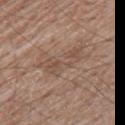Assessment: The lesion was photographed on a routine skin check and not biopsied; there is no pathology result. Context: A 15 mm close-up tile from a total-body photography series done for melanoma screening. The subject is a male aged 53 to 57. Measured at roughly 5.5 mm in maximum diameter. Located on the leg.